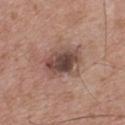Clinical impression:
Imaged during a routine full-body skin examination; the lesion was not biopsied and no histopathology is available.
Background:
The total-body-photography lesion software estimated an area of roughly 14 mm², an eccentricity of roughly 0.75, and a shape-asymmetry score of about 0.15 (0 = symmetric). It also reported a lesion color around L≈47 a*≈18 b*≈23 in CIELAB, roughly 13 lightness units darker than nearby skin, and a normalized border contrast of about 9.5. The software also gave border irregularity of about 2 on a 0–10 scale, a color-variation rating of about 8/10, and a peripheral color-asymmetry measure near 2.5. This is a white-light tile. About 5 mm across. This image is a 15 mm lesion crop taken from a total-body photograph. A male patient, about 65 years old. The lesion is located on the upper back.